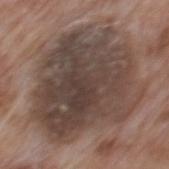Clinical summary:
A male patient in their 70s. The lesion is located on the mid back. Imaged with white-light lighting. The lesion's longest dimension is about 10.5 mm. A close-up tile cropped from a whole-body skin photograph, about 15 mm across.